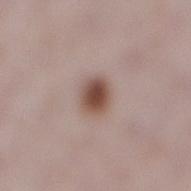Q: Is there a histopathology result?
A: imaged on a skin check; not biopsied
Q: What is the imaging modality?
A: total-body-photography crop, ~15 mm field of view
Q: Where on the body is the lesion?
A: the right lower leg
Q: Who is the patient?
A: female, roughly 30 years of age
Q: What lighting was used for the tile?
A: white-light illumination
Q: How large is the lesion?
A: about 3 mm